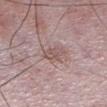workup — total-body-photography surveillance lesion; no biopsy | anatomic site — the leg | automated lesion analysis — a lesion area of about 4 mm², a shape eccentricity near 0.75, and a symmetry-axis asymmetry near 0.3; a border-irregularity rating of about 2.5/10, a color-variation rating of about 3/10, and a peripheral color-asymmetry measure near 1; an automated nevus-likeness rating near 0 out of 100 and lesion-presence confidence of about 90/100 | subject — male, approximately 50 years of age | acquisition — ~15 mm tile from a whole-body skin photo | lighting — white-light illumination | lesion size — about 2.5 mm.